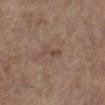Q: Is there a histopathology result?
A: total-body-photography surveillance lesion; no biopsy
Q: How large is the lesion?
A: about 3 mm
Q: Who is the patient?
A: female, in their mid- to late 60s
Q: What kind of image is this?
A: ~15 mm crop, total-body skin-cancer survey
Q: Lesion location?
A: the left lower leg
Q: What did automated image analysis measure?
A: a lesion–skin lightness drop of about 7 and a normalized border contrast of about 5.5